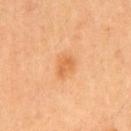Assessment: Captured during whole-body skin photography for melanoma surveillance; the lesion was not biopsied. Background: Located on the chest. Cropped from a total-body skin-imaging series; the visible field is about 15 mm. A male subject, approximately 60 years of age. The total-body-photography lesion software estimated a lesion area of about 4.5 mm² and a shape-asymmetry score of about 0.3 (0 = symmetric). Captured under cross-polarized illumination. The lesion's longest dimension is about 2.5 mm.